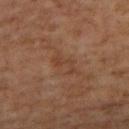No biopsy was performed on this lesion — it was imaged during a full skin examination and was not determined to be concerning. Imaged with cross-polarized lighting. The recorded lesion diameter is about 3.5 mm. A female patient aged approximately 60. A lesion tile, about 15 mm wide, cut from a 3D total-body photograph. The lesion is located on the upper back.